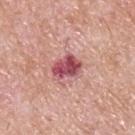Part of a total-body skin-imaging series; this lesion was reviewed on a skin check and was not flagged for biopsy.